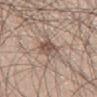notes: imaged on a skin check; not biopsied
patient: male, aged approximately 45
anatomic site: the left thigh
size: about 4 mm
automated metrics: a mean CIELAB color near L≈52 a*≈15 b*≈24, a lesion–skin lightness drop of about 11, and a normalized lesion–skin contrast near 8
imaging modality: total-body-photography crop, ~15 mm field of view